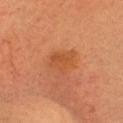notes: imaged on a skin check; not biopsied
diameter: ~3 mm (longest diameter)
subject: female, in their 30s
location: the head or neck
lighting: cross-polarized
TBP lesion metrics: an area of roughly 6.5 mm², a shape eccentricity near 0.5, and a symmetry-axis asymmetry near 0.35; roughly 6 lightness units darker than nearby skin and a lesion-to-skin contrast of about 5.5 (normalized; higher = more distinct); a border-irregularity rating of about 3.5/10, a color-variation rating of about 2/10, and a peripheral color-asymmetry measure near 0.5; an automated nevus-likeness rating near 5 out of 100 and a lesion-detection confidence of about 100/100
image source: total-body-photography crop, ~15 mm field of view